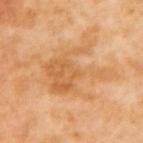notes = total-body-photography surveillance lesion; no biopsy
subject = female, approximately 55 years of age
lighting = cross-polarized illumination
image = ~15 mm tile from a whole-body skin photo
anatomic site = the upper back